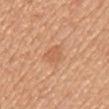The lesion was photographed on a routine skin check and not biopsied; there is no pathology result. The lesion is located on the chest. The total-body-photography lesion software estimated a lesion-detection confidence of about 100/100. A female subject, aged around 40. A region of skin cropped from a whole-body photographic capture, roughly 15 mm wide. The lesion's longest dimension is about 3 mm.